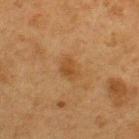| feature | finding |
|---|---|
| notes | total-body-photography surveillance lesion; no biopsy |
| image-analysis metrics | an area of roughly 4.5 mm², an outline eccentricity of about 0.6 (0 = round, 1 = elongated), and two-axis asymmetry of about 0.3; border irregularity of about 2.5 on a 0–10 scale, internal color variation of about 1.5 on a 0–10 scale, and radial color variation of about 0.5 |
| illumination | cross-polarized |
| site | the upper back |
| lesion size | ≈2.5 mm |
| acquisition | 15 mm crop, total-body photography |
| subject | male, in their mid-70s |